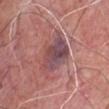Case summary:
* notes: imaged on a skin check; not biopsied
* location: the chest
* patient: male, in their 60s
* acquisition: ~15 mm tile from a whole-body skin photo